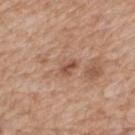Part of a total-body skin-imaging series; this lesion was reviewed on a skin check and was not flagged for biopsy. The lesion is located on the mid back. A male subject, aged 58–62. A close-up tile cropped from a whole-body skin photograph, about 15 mm across. Longest diameter approximately 3 mm. The tile uses white-light illumination.